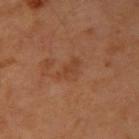From the arm.
A close-up tile cropped from a whole-body skin photograph, about 15 mm across.
The total-body-photography lesion software estimated a footprint of about 4 mm² and a symmetry-axis asymmetry near 0.4. And it measured a lesion color around L≈40 a*≈23 b*≈32 in CIELAB, a lesion–skin lightness drop of about 5, and a normalized lesion–skin contrast near 5. And it measured a border-irregularity rating of about 4.5/10 and a color-variation rating of about 1/10.
The patient is a male in their mid- to late 60s.